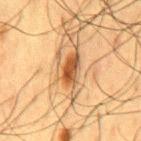The lesion was tiled from a total-body skin photograph and was not biopsied.
Measured at roughly 4 mm in maximum diameter.
The lesion is on the mid back.
Imaged with cross-polarized lighting.
The subject is a male roughly 60 years of age.
Cropped from a whole-body photographic skin survey; the tile spans about 15 mm.
An algorithmic analysis of the crop reported an area of roughly 8 mm² and two-axis asymmetry of about 0.35.